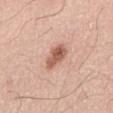Recorded during total-body skin imaging; not selected for excision or biopsy.
A male subject, approximately 60 years of age.
The lesion's longest dimension is about 3.5 mm.
Imaged with white-light lighting.
On the mid back.
The total-body-photography lesion software estimated an outline eccentricity of about 0.8 (0 = round, 1 = elongated). And it measured a border-irregularity index near 2.5/10 and a peripheral color-asymmetry measure near 1.5. And it measured a nevus-likeness score of about 90/100.
A 15 mm crop from a total-body photograph taken for skin-cancer surveillance.